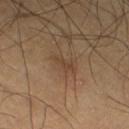{"biopsy_status": "not biopsied; imaged during a skin examination", "site": "right thigh", "patient": {"sex": "male", "age_approx": 60}, "image": {"source": "total-body photography crop", "field_of_view_mm": 15}}